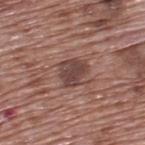No biopsy was performed on this lesion — it was imaged during a full skin examination and was not determined to be concerning. This is a white-light tile. A 15 mm close-up tile from a total-body photography series done for melanoma screening. About 3.5 mm across. From the back. A male patient in their 70s.